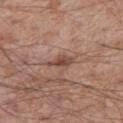Assessment: Part of a total-body skin-imaging series; this lesion was reviewed on a skin check and was not flagged for biopsy. Clinical summary: The tile uses white-light illumination. Cropped from a whole-body photographic skin survey; the tile spans about 15 mm. Approximately 3 mm at its widest. The patient is a male approximately 55 years of age. Located on the right thigh.